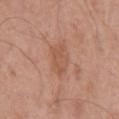Clinical impression: Imaged during a routine full-body skin examination; the lesion was not biopsied and no histopathology is available. Acquisition and patient details: Imaged with white-light lighting. A 15 mm crop from a total-body photograph taken for skin-cancer surveillance. A male subject, approximately 70 years of age. The lesion is located on the left upper arm.